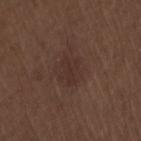workup: imaged on a skin check; not biopsied | lighting: white-light illumination | subject: male, in their 70s | automated lesion analysis: an area of roughly 6 mm² and an eccentricity of roughly 0.7; a lesion color around L≈30 a*≈16 b*≈21 in CIELAB, a lesion–skin lightness drop of about 5, and a normalized lesion–skin contrast near 5.5; border irregularity of about 3.5 on a 0–10 scale and a peripheral color-asymmetry measure near 0.5 | imaging modality: 15 mm crop, total-body photography | site: the back.